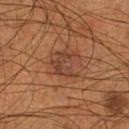Recorded during total-body skin imaging; not selected for excision or biopsy.
The lesion is located on the leg.
A male patient, in their mid- to late 50s.
A roughly 15 mm field-of-view crop from a total-body skin photograph.
The tile uses cross-polarized illumination.